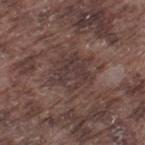Part of a total-body skin-imaging series; this lesion was reviewed on a skin check and was not flagged for biopsy. The patient is a male in their mid-70s. The lesion is on the leg. A region of skin cropped from a whole-body photographic capture, roughly 15 mm wide.